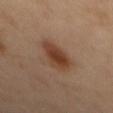Notes:
– workup: catalogued during a skin exam; not biopsied
– image source: ~15 mm crop, total-body skin-cancer survey
– body site: the mid back
– lesion diameter: ≈5 mm
– tile lighting: cross-polarized illumination
– image-analysis metrics: a lesion area of about 10 mm² and a shape-asymmetry score of about 0.2 (0 = symmetric); a lesion–skin lightness drop of about 11 and a lesion-to-skin contrast of about 9.5 (normalized; higher = more distinct); border irregularity of about 2.5 on a 0–10 scale and peripheral color asymmetry of about 1
– subject: male, aged approximately 55